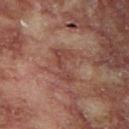<lesion>
<site>chest</site>
<lighting>cross-polarized</lighting>
<image>
  <source>total-body photography crop</source>
  <field_of_view_mm>15</field_of_view_mm>
</image>
<automated_metrics>
  <area_mm2_approx>6.5</area_mm2_approx>
  <shape_asymmetry>0.5</shape_asymmetry>
  <cielab_L>38</cielab_L>
  <cielab_a>21</cielab_a>
  <cielab_b>22</cielab_b>
  <vs_skin_darker_L>7.0</vs_skin_darker_L>
  <vs_skin_contrast_norm>6.0</vs_skin_contrast_norm>
  <nevus_likeness_0_100>0</nevus_likeness_0_100>
  <lesion_detection_confidence_0_100>100</lesion_detection_confidence_0_100>
</automated_metrics>
<patient>
  <sex>male</sex>
  <age_approx>55</age_approx>
</patient>
</lesion>